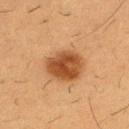follow-up = no biopsy performed (imaged during a skin exam) | illumination = cross-polarized illumination | image source = 15 mm crop, total-body photography | automated metrics = a shape eccentricity near 0.7 and a symmetry-axis asymmetry near 0.35; a border-irregularity index near 5/10, internal color variation of about 7 on a 0–10 scale, and peripheral color asymmetry of about 1.5 | body site = the front of the torso | patient = male, aged 53 to 57.